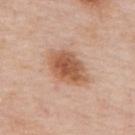<tbp_lesion>
<biopsy_status>not biopsied; imaged during a skin examination</biopsy_status>
<patient>
  <sex>male</sex>
  <age_approx>75</age_approx>
</patient>
<site>upper back</site>
<lighting>white-light</lighting>
<image>
  <source>total-body photography crop</source>
  <field_of_view_mm>15</field_of_view_mm>
</image>
<lesion_size>
  <long_diameter_mm_approx>5.5</long_diameter_mm_approx>
</lesion_size>
</tbp_lesion>